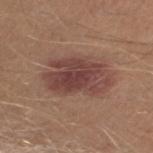Imaged during a routine full-body skin examination; the lesion was not biopsied and no histopathology is available.
A male patient, aged around 30.
Cropped from a whole-body photographic skin survey; the tile spans about 15 mm.
The lesion is located on the leg.